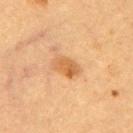The lesion was tiled from a total-body skin photograph and was not biopsied. The lesion's longest dimension is about 3.5 mm. The tile uses cross-polarized illumination. Located on the upper back. A 15 mm close-up tile from a total-body photography series done for melanoma screening. A female subject, aged 58 to 62. An algorithmic analysis of the crop reported a within-lesion color-variation index near 4/10.